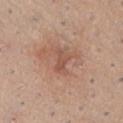image: ~15 mm tile from a whole-body skin photo; anatomic site: the abdomen; lesion diameter: ~2.5 mm (longest diameter); patient: male, aged 38 to 42.